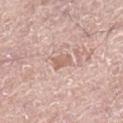Notes:
* workup — no biopsy performed (imaged during a skin exam)
* body site — the right lower leg
* subject — male, aged 58–62
* image source — total-body-photography crop, ~15 mm field of view